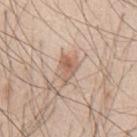Notes:
• notes — catalogued during a skin exam; not biopsied
• diameter — ≈3.5 mm
• location — the mid back
• subject — male, in their mid- to late 40s
• acquisition — 15 mm crop, total-body photography
• lighting — white-light